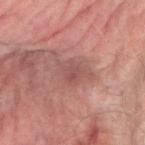Clinical impression: No biopsy was performed on this lesion — it was imaged during a full skin examination and was not determined to be concerning. Context: Measured at roughly 2.5 mm in maximum diameter. A region of skin cropped from a whole-body photographic capture, roughly 15 mm wide. On the right forearm. A male patient, about 75 years old.